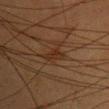The lesion was photographed on a routine skin check and not biopsied; there is no pathology result. An algorithmic analysis of the crop reported a footprint of about 4 mm², a shape eccentricity near 0.85, and a shape-asymmetry score of about 0.45 (0 = symmetric). It also reported a color-variation rating of about 1.5/10 and peripheral color asymmetry of about 0.5. On the chest. The patient is a female aged around 55. Longest diameter approximately 3 mm. Cropped from a whole-body photographic skin survey; the tile spans about 15 mm.